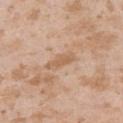Impression:
The lesion was photographed on a routine skin check and not biopsied; there is no pathology result.
Clinical summary:
A female subject aged around 25. The lesion-visualizer software estimated a footprint of about 5 mm² and an eccentricity of roughly 0.9. And it measured a border-irregularity index near 3.5/10, a within-lesion color-variation index near 1.5/10, and radial color variation of about 0.5. And it measured a nevus-likeness score of about 0/100 and a lesion-detection confidence of about 90/100. Captured under white-light illumination. A lesion tile, about 15 mm wide, cut from a 3D total-body photograph. The lesion is on the left upper arm. The lesion's longest dimension is about 4 mm.